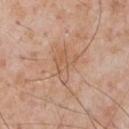workup=no biopsy performed (imaged during a skin exam)
diameter=about 4 mm
imaging modality=15 mm crop, total-body photography
lighting=white-light
location=the front of the torso
subject=male, roughly 60 years of age